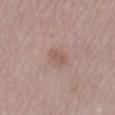- follow-up · no biopsy performed (imaged during a skin exam)
- diameter · ≈3 mm
- tile lighting · white-light
- TBP lesion metrics · a lesion area of about 4.5 mm², an outline eccentricity of about 0.75 (0 = round, 1 = elongated), and a symmetry-axis asymmetry near 0.2; about 7 CIELAB-L* units darker than the surrounding skin
- location · the right lower leg
- patient · female, roughly 40 years of age
- acquisition · ~15 mm tile from a whole-body skin photo